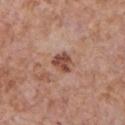Q: Is there a histopathology result?
A: total-body-photography surveillance lesion; no biopsy
Q: What lighting was used for the tile?
A: white-light
Q: What is the anatomic site?
A: the front of the torso
Q: Patient demographics?
A: male, aged approximately 65
Q: How was this image acquired?
A: total-body-photography crop, ~15 mm field of view